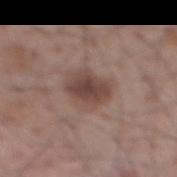{"image": {"source": "total-body photography crop", "field_of_view_mm": 15}, "site": "mid back", "patient": {"sex": "male", "age_approx": 70}}